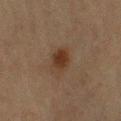| feature | finding |
|---|---|
| follow-up | no biopsy performed (imaged during a skin exam) |
| location | the right upper arm |
| patient | male, aged 63 to 67 |
| acquisition | 15 mm crop, total-body photography |